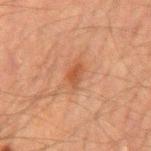Imaged during a routine full-body skin examination; the lesion was not biopsied and no histopathology is available.
On the mid back.
Imaged with cross-polarized lighting.
The patient is a male aged approximately 60.
The recorded lesion diameter is about 3 mm.
Cropped from a whole-body photographic skin survey; the tile spans about 15 mm.